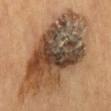Assessment:
This lesion was catalogued during total-body skin photography and was not selected for biopsy.
Image and clinical context:
The lesion is located on the abdomen. This is a cross-polarized tile. Cropped from a whole-body photographic skin survey; the tile spans about 15 mm. A male patient, aged around 55.